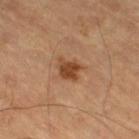follow-up: total-body-photography surveillance lesion; no biopsy
lighting: cross-polarized
location: the right thigh
patient: male, aged 58 to 62
size: ~3 mm (longest diameter)
automated lesion analysis: a border-irregularity index near 3.5/10 and a color-variation rating of about 2/10; a classifier nevus-likeness of about 85/100 and lesion-presence confidence of about 100/100
image source: total-body-photography crop, ~15 mm field of view